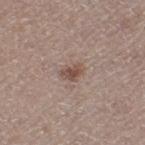Context:
The lesion's longest dimension is about 2.5 mm. A female subject, roughly 50 years of age. A lesion tile, about 15 mm wide, cut from a 3D total-body photograph. On the left thigh. Imaged with white-light lighting.